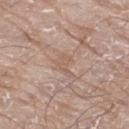This is a white-light tile.
A male patient, aged approximately 80.
A 15 mm close-up extracted from a 3D total-body photography capture.
Located on the left leg.
Approximately 2.5 mm at its widest.